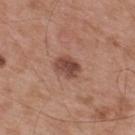Q: Was a biopsy performed?
A: no biopsy performed (imaged during a skin exam)
Q: What are the patient's age and sex?
A: male, approximately 55 years of age
Q: Lesion location?
A: the back
Q: How was this image acquired?
A: 15 mm crop, total-body photography